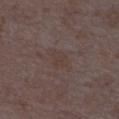subject: female, aged 33–37; location: the left lower leg; acquisition: ~15 mm tile from a whole-body skin photo; lighting: white-light illumination; TBP lesion metrics: a border-irregularity index near 2/10 and radial color variation of about 0.5; lesion diameter: ~3 mm (longest diameter).